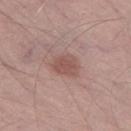{"biopsy_status": "not biopsied; imaged during a skin examination", "lesion_size": {"long_diameter_mm_approx": 3.0}, "image": {"source": "total-body photography crop", "field_of_view_mm": 15}, "lighting": "white-light", "site": "left thigh", "patient": {"sex": "male", "age_approx": 55}}